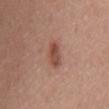Captured during whole-body skin photography for melanoma surveillance; the lesion was not biopsied.
About 3.5 mm across.
The lesion is located on the mid back.
The subject is a male roughly 55 years of age.
The total-body-photography lesion software estimated a lesion area of about 5.5 mm² and an eccentricity of roughly 0.85. And it measured a border-irregularity rating of about 2.5/10, internal color variation of about 3.5 on a 0–10 scale, and radial color variation of about 1. The software also gave an automated nevus-likeness rating near 90 out of 100 and a lesion-detection confidence of about 100/100.
A 15 mm close-up tile from a total-body photography series done for melanoma screening.
Imaged with white-light lighting.